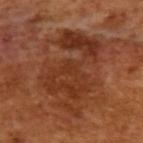biopsy status: total-body-photography surveillance lesion; no biopsy | patient: male, approximately 65 years of age | image source: 15 mm crop, total-body photography | image-analysis metrics: an eccentricity of roughly 0.85; an average lesion color of about L≈34 a*≈24 b*≈32 (CIELAB), a lesion–skin lightness drop of about 8, and a normalized lesion–skin contrast near 7.5; a classifier nevus-likeness of about 0/100 and lesion-presence confidence of about 95/100 | lesion size: about 9 mm | illumination: cross-polarized.